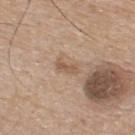<case>
<biopsy_status>not biopsied; imaged during a skin examination</biopsy_status>
<automated_metrics>
  <cielab_L>56</cielab_L>
  <cielab_a>17</cielab_a>
  <cielab_b>31</cielab_b>
  <border_irregularity_0_10>3.0</border_irregularity_0_10>
  <color_variation_0_10>2.0</color_variation_0_10>
  <peripheral_color_asymmetry>0.5</peripheral_color_asymmetry>
  <nevus_likeness_0_100>0</nevus_likeness_0_100>
  <lesion_detection_confidence_0_100>100</lesion_detection_confidence_0_100>
</automated_metrics>
<lighting>white-light</lighting>
<lesion_size>
  <long_diameter_mm_approx>2.5</long_diameter_mm_approx>
</lesion_size>
<patient>
  <sex>male</sex>
  <age_approx>75</age_approx>
</patient>
<site>upper back</site>
<image>
  <source>total-body photography crop</source>
  <field_of_view_mm>15</field_of_view_mm>
</image>
</case>